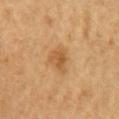Q: Is there a histopathology result?
A: imaged on a skin check; not biopsied
Q: How was this image acquired?
A: ~15 mm crop, total-body skin-cancer survey
Q: What is the anatomic site?
A: the right upper arm
Q: How was the tile lit?
A: cross-polarized
Q: Lesion size?
A: ≈3.5 mm
Q: What did automated image analysis measure?
A: an average lesion color of about L≈52 a*≈19 b*≈39 (CIELAB), roughly 9 lightness units darker than nearby skin, and a normalized lesion–skin contrast near 6.5; internal color variation of about 3 on a 0–10 scale and peripheral color asymmetry of about 1; a nevus-likeness score of about 55/100 and a detector confidence of about 100 out of 100 that the crop contains a lesion
Q: Patient demographics?
A: female, aged 58 to 62